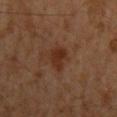Background: On the mid back. A male subject aged 58 to 62. A region of skin cropped from a whole-body photographic capture, roughly 15 mm wide. Approximately 3.5 mm at its widest.